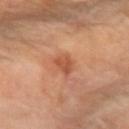Captured during whole-body skin photography for melanoma surveillance; the lesion was not biopsied. The recorded lesion diameter is about 2.5 mm. A roughly 15 mm field-of-view crop from a total-body skin photograph. The total-body-photography lesion software estimated a footprint of about 4 mm², an outline eccentricity of about 0.5 (0 = round, 1 = elongated), and two-axis asymmetry of about 0.3. It also reported a classifier nevus-likeness of about 25/100 and a lesion-detection confidence of about 100/100. The patient is a female aged 68–72. The lesion is on the left forearm.